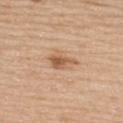Clinical impression: The lesion was photographed on a routine skin check and not biopsied; there is no pathology result. Background: A female patient approximately 65 years of age. Captured under white-light illumination. A 15 mm close-up tile from a total-body photography series done for melanoma screening. From the upper back. An algorithmic analysis of the crop reported border irregularity of about 4 on a 0–10 scale and internal color variation of about 5.5 on a 0–10 scale. About 3.5 mm across.